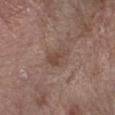Imaged during a routine full-body skin examination; the lesion was not biopsied and no histopathology is available. From the arm. A region of skin cropped from a whole-body photographic capture, roughly 15 mm wide. The recorded lesion diameter is about 3.5 mm. A male subject in their 70s. The total-body-photography lesion software estimated a lesion color around L≈45 a*≈17 b*≈23 in CIELAB and a lesion-to-skin contrast of about 5.5 (normalized; higher = more distinct). And it measured an automated nevus-likeness rating near 0 out of 100 and a lesion-detection confidence of about 100/100.